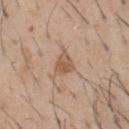Assessment:
This lesion was catalogued during total-body skin photography and was not selected for biopsy.
Clinical summary:
Cropped from a total-body skin-imaging series; the visible field is about 15 mm. A male subject, in their 60s. An algorithmic analysis of the crop reported an area of roughly 5.5 mm², an outline eccentricity of about 0.6 (0 = round, 1 = elongated), and a symmetry-axis asymmetry near 0.3. It also reported a border-irregularity index near 3/10, a within-lesion color-variation index near 4.5/10, and radial color variation of about 1.5. And it measured a nevus-likeness score of about 45/100. Located on the chest. Longest diameter approximately 3 mm. This is a white-light tile.